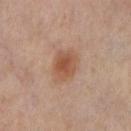No biopsy was performed on this lesion — it was imaged during a full skin examination and was not determined to be concerning.
A 15 mm crop from a total-body photograph taken for skin-cancer surveillance.
The lesion is located on the left thigh.
This is a cross-polarized tile.
A female subject aged around 50.
The lesion-visualizer software estimated a footprint of about 8.5 mm² and an eccentricity of roughly 0.7. The software also gave an average lesion color of about L≈50 a*≈20 b*≈30 (CIELAB), about 9 CIELAB-L* units darker than the surrounding skin, and a normalized border contrast of about 7.5. The analysis additionally found border irregularity of about 1.5 on a 0–10 scale and peripheral color asymmetry of about 1.